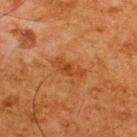Imaged during a routine full-body skin examination; the lesion was not biopsied and no histopathology is available.
Cropped from a whole-body photographic skin survey; the tile spans about 15 mm.
About 4 mm across.
A male subject aged 63–67.
Automated image analysis of the tile measured a mean CIELAB color near L≈38 a*≈24 b*≈35, a lesion–skin lightness drop of about 7, and a normalized border contrast of about 6.5. The software also gave a border-irregularity index near 3.5/10, a color-variation rating of about 2/10, and radial color variation of about 0.5.
On the back.
This is a cross-polarized tile.